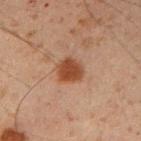Longest diameter approximately 3 mm. A lesion tile, about 15 mm wide, cut from a 3D total-body photograph. The subject is a male aged around 50. Captured under cross-polarized illumination. On the right upper arm.